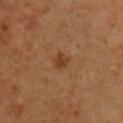* biopsy status: no biopsy performed (imaged during a skin exam)
* TBP lesion metrics: a mean CIELAB color near L≈41 a*≈22 b*≈36, a lesion–skin lightness drop of about 8, and a normalized border contrast of about 7.5; border irregularity of about 2.5 on a 0–10 scale; a nevus-likeness score of about 60/100 and a lesion-detection confidence of about 100/100
* lighting: cross-polarized illumination
* patient: female, aged around 55
* image: ~15 mm crop, total-body skin-cancer survey
* body site: the chest